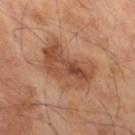Notes:
– biopsy status: total-body-photography surveillance lesion; no biopsy
– automated metrics: an eccentricity of roughly 0.8 and a symmetry-axis asymmetry near 0.3; a lesion color around L≈48 a*≈22 b*≈32 in CIELAB, a lesion–skin lightness drop of about 10, and a normalized lesion–skin contrast near 7; a border-irregularity index near 4/10, a within-lesion color-variation index near 6.5/10, and a peripheral color-asymmetry measure near 2.5; a classifier nevus-likeness of about 35/100
– image: 15 mm crop, total-body photography
– diameter: ~7 mm (longest diameter)
– anatomic site: the right thigh
– subject: male, aged around 70